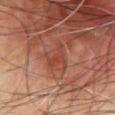<record>
<site>chest</site>
<patient>
  <sex>male</sex>
  <age_approx>65</age_approx>
</patient>
<image>
  <source>total-body photography crop</source>
  <field_of_view_mm>15</field_of_view_mm>
</image>
</record>